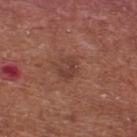workup=imaged on a skin check; not biopsied | anatomic site=the upper back | subject=male, aged around 65 | acquisition=~15 mm tile from a whole-body skin photo | lesion size=~2.5 mm (longest diameter).